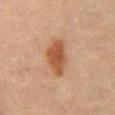Part of a total-body skin-imaging series; this lesion was reviewed on a skin check and was not flagged for biopsy.
A region of skin cropped from a whole-body photographic capture, roughly 15 mm wide.
A male patient, aged around 65.
On the mid back.